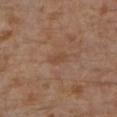workup: imaged on a skin check; not biopsied
automated metrics: a footprint of about 3 mm², a shape eccentricity near 0.85, and a symmetry-axis asymmetry near 0.2; a lesion color around L≈44 a*≈19 b*≈29 in CIELAB, a lesion–skin lightness drop of about 5, and a lesion-to-skin contrast of about 5 (normalized; higher = more distinct); a border-irregularity index near 2/10; an automated nevus-likeness rating near 0 out of 100 and a detector confidence of about 100 out of 100 that the crop contains a lesion
subject: male, in their 30s
image: ~15 mm crop, total-body skin-cancer survey
diameter: ≈2.5 mm
illumination: cross-polarized illumination
body site: the left lower leg